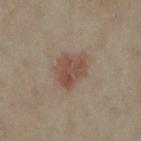{"biopsy_status": "not biopsied; imaged during a skin examination", "lighting": "cross-polarized", "image": {"source": "total-body photography crop", "field_of_view_mm": 15}, "lesion_size": {"long_diameter_mm_approx": 4.0}, "automated_metrics": {"area_mm2_approx": 10.0, "shape_asymmetry": 0.3, "border_irregularity_0_10": 3.0, "color_variation_0_10": 3.5, "peripheral_color_asymmetry": 1.0, "nevus_likeness_0_100": 95, "lesion_detection_confidence_0_100": 100}, "site": "leg", "patient": {"sex": "female", "age_approx": 35}}